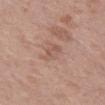Notes:
- notes — catalogued during a skin exam; not biopsied
- patient — female, aged approximately 40
- automated lesion analysis — an area of roughly 5 mm² and a shape-asymmetry score of about 0.3 (0 = symmetric); a normalized border contrast of about 5
- lighting — white-light illumination
- lesion size — ≈3.5 mm
- image source — total-body-photography crop, ~15 mm field of view
- body site — the abdomen